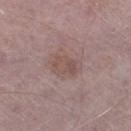Impression: Recorded during total-body skin imaging; not selected for excision or biopsy. Image and clinical context: A 15 mm close-up extracted from a 3D total-body photography capture. The tile uses white-light illumination. An algorithmic analysis of the crop reported a border-irregularity index near 3.5/10, a color-variation rating of about 3.5/10, and radial color variation of about 1.5. About 3 mm across. A male subject approximately 75 years of age. The lesion is located on the left thigh.